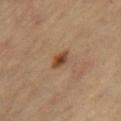Q: Is there a histopathology result?
A: imaged on a skin check; not biopsied
Q: What is the imaging modality?
A: 15 mm crop, total-body photography
Q: Who is the patient?
A: female, aged 63 to 67
Q: What is the anatomic site?
A: the right thigh